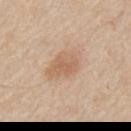Clinical impression:
The lesion was photographed on a routine skin check and not biopsied; there is no pathology result.
Acquisition and patient details:
Captured under white-light illumination. From the mid back. The patient is a male aged 58 to 62. Measured at roughly 6 mm in maximum diameter. A region of skin cropped from a whole-body photographic capture, roughly 15 mm wide.